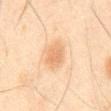Captured during whole-body skin photography for melanoma surveillance; the lesion was not biopsied. A region of skin cropped from a whole-body photographic capture, roughly 15 mm wide. A male subject approximately 45 years of age. Longest diameter approximately 3 mm. The lesion is on the abdomen.